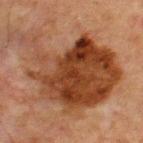notes: catalogued during a skin exam; not biopsied